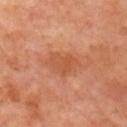notes: total-body-photography surveillance lesion; no biopsy | acquisition: 15 mm crop, total-body photography | anatomic site: the right upper arm | subject: male, approximately 70 years of age.